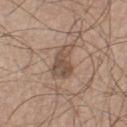Notes:
• workup — catalogued during a skin exam; not biopsied
• image source — 15 mm crop, total-body photography
• illumination — white-light illumination
• lesion size — ≈4.5 mm
• subject — male, aged 73 to 77
• automated metrics — border irregularity of about 4 on a 0–10 scale, a within-lesion color-variation index near 4.5/10, and peripheral color asymmetry of about 1.5
• site — the left thigh